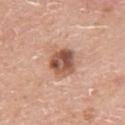follow-up=total-body-photography surveillance lesion; no biopsy
tile lighting=white-light
location=the upper back
patient=male, aged approximately 60
size=~3.5 mm (longest diameter)
image=~15 mm tile from a whole-body skin photo